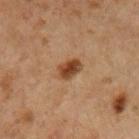No biopsy was performed on this lesion — it was imaged during a full skin examination and was not determined to be concerning. A region of skin cropped from a whole-body photographic capture, roughly 15 mm wide. Located on the right upper arm. The lesion-visualizer software estimated an area of roughly 5 mm², an eccentricity of roughly 0.6, and a shape-asymmetry score of about 0.2 (0 = symmetric). And it measured an average lesion color of about L≈33 a*≈17 b*≈27 (CIELAB), roughly 11 lightness units darker than nearby skin, and a normalized border contrast of about 10. The software also gave internal color variation of about 4 on a 0–10 scale. The tile uses cross-polarized illumination. A male patient about 60 years old.